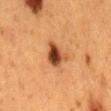Impression:
The lesion was tiled from a total-body skin photograph and was not biopsied.
Acquisition and patient details:
Located on the mid back. A region of skin cropped from a whole-body photographic capture, roughly 15 mm wide. An algorithmic analysis of the crop reported an area of roughly 6.5 mm², an outline eccentricity of about 0.7 (0 = round, 1 = elongated), and two-axis asymmetry of about 0.45. And it measured an average lesion color of about L≈38 a*≈23 b*≈33 (CIELAB) and roughly 16 lightness units darker than nearby skin. It also reported a border-irregularity rating of about 4/10 and radial color variation of about 2. A female subject, aged 48–52. Captured under cross-polarized illumination.